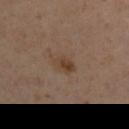Impression: Recorded during total-body skin imaging; not selected for excision or biopsy. Clinical summary: This image is a 15 mm lesion crop taken from a total-body photograph. On the left upper arm. A male subject, about 45 years old. Imaged with cross-polarized lighting.